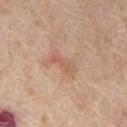This lesion was catalogued during total-body skin photography and was not selected for biopsy.
A male patient approximately 65 years of age.
From the front of the torso.
A lesion tile, about 15 mm wide, cut from a 3D total-body photograph.
The recorded lesion diameter is about 3.5 mm.
This is a white-light tile.